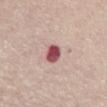Findings:
* workup — no biopsy performed (imaged during a skin exam)
* subject — female, aged around 70
* body site — the abdomen
* tile lighting — white-light illumination
* image source — ~15 mm tile from a whole-body skin photo
* diameter — ≈2.5 mm